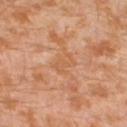follow-up = total-body-photography surveillance lesion; no biopsy
image = 15 mm crop, total-body photography
subject = male, aged approximately 30
body site = the left lower leg